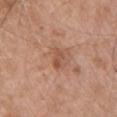Assessment:
Recorded during total-body skin imaging; not selected for excision or biopsy.
Context:
The patient is a male aged 63 to 67. Located on the chest. A region of skin cropped from a whole-body photographic capture, roughly 15 mm wide. Measured at roughly 2.5 mm in maximum diameter. The tile uses white-light illumination.